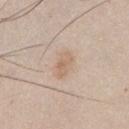notes: no biopsy performed (imaged during a skin exam) | body site: the chest | patient: male, approximately 45 years of age | acquisition: ~15 mm tile from a whole-body skin photo.